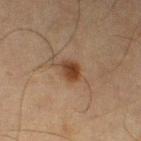– follow-up: catalogued during a skin exam; not biopsied